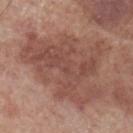notes=catalogued during a skin exam; not biopsied | subject=male, in their 70s | image source=~15 mm tile from a whole-body skin photo | anatomic site=the right lower leg.